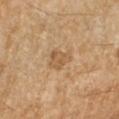Part of a total-body skin-imaging series; this lesion was reviewed on a skin check and was not flagged for biopsy. Cropped from a total-body skin-imaging series; the visible field is about 15 mm. The subject is a female approximately 70 years of age. Located on the right forearm. The lesion's longest dimension is about 3 mm. This is a cross-polarized tile.